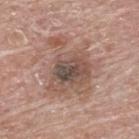<record>
<biopsy_status>not biopsied; imaged during a skin examination</biopsy_status>
<site>back</site>
<image>
  <source>total-body photography crop</source>
  <field_of_view_mm>15</field_of_view_mm>
</image>
<lesion_size>
  <long_diameter_mm_approx>7.0</long_diameter_mm_approx>
</lesion_size>
<patient>
  <sex>male</sex>
  <age_approx>85+</age_approx>
</patient>
</record>